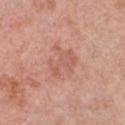Notes:
- notes: total-body-photography surveillance lesion; no biopsy
- acquisition: ~15 mm crop, total-body skin-cancer survey
- illumination: white-light illumination
- patient: female, about 55 years old
- size: ~3.5 mm (longest diameter)
- TBP lesion metrics: an average lesion color of about L≈58 a*≈25 b*≈29 (CIELAB), roughly 7 lightness units darker than nearby skin, and a normalized border contrast of about 5
- site: the chest